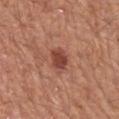Part of a total-body skin-imaging series; this lesion was reviewed on a skin check and was not flagged for biopsy. A lesion tile, about 15 mm wide, cut from a 3D total-body photograph. On the mid back. An algorithmic analysis of the crop reported an eccentricity of roughly 0.6 and two-axis asymmetry of about 0.25. The tile uses white-light illumination. A male subject, roughly 65 years of age. Measured at roughly 2.5 mm in maximum diameter.